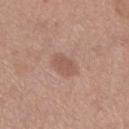patient=female, in their mid-50s; image source=~15 mm tile from a whole-body skin photo; body site=the leg; diameter=≈3 mm; tile lighting=white-light.